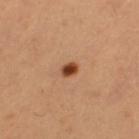biopsy status = catalogued during a skin exam; not biopsied | subject = female, in their mid- to late 50s | site = the left thigh | acquisition = 15 mm crop, total-body photography.